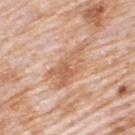{"patient": {"sex": "male", "age_approx": 80}, "lesion_size": {"long_diameter_mm_approx": 6.0}, "automated_metrics": {"area_mm2_approx": 12.0, "eccentricity": 0.9, "shape_asymmetry": 0.35}, "site": "upper back", "lighting": "white-light", "image": {"source": "total-body photography crop", "field_of_view_mm": 15}}